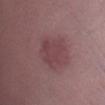No biopsy was performed on this lesion — it was imaged during a full skin examination and was not determined to be concerning.
Cropped from a whole-body photographic skin survey; the tile spans about 15 mm.
The subject is a male in their 30s.
This is a white-light tile.
Measured at roughly 4 mm in maximum diameter.